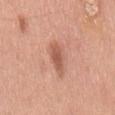<tbp_lesion>
  <biopsy_status>not biopsied; imaged during a skin examination</biopsy_status>
  <patient>
    <sex>male</sex>
    <age_approx>50</age_approx>
  </patient>
  <image>
    <source>total-body photography crop</source>
    <field_of_view_mm>15</field_of_view_mm>
  </image>
  <site>mid back</site>
</tbp_lesion>